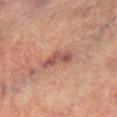Part of a total-body skin-imaging series; this lesion was reviewed on a skin check and was not flagged for biopsy. About 4.5 mm across. The lesion is located on the right lower leg. Cropped from a whole-body photographic skin survey; the tile spans about 15 mm. The total-body-photography lesion software estimated an area of roughly 6 mm² and an outline eccentricity of about 0.95 (0 = round, 1 = elongated). A male subject aged 73 to 77. The tile uses cross-polarized illumination.